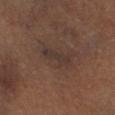Captured during whole-body skin photography for melanoma surveillance; the lesion was not biopsied.
The lesion's longest dimension is about 4 mm.
The lesion is located on the left lower leg.
Captured under cross-polarized illumination.
The subject is a female aged 63 to 67.
A 15 mm close-up extracted from a 3D total-body photography capture.